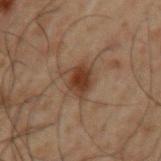Recorded during total-body skin imaging; not selected for excision or biopsy. This image is a 15 mm lesion crop taken from a total-body photograph. The lesion is on the left upper arm. The tile uses cross-polarized illumination. A male subject about 50 years old. An algorithmic analysis of the crop reported a nevus-likeness score of about 85/100 and a lesion-detection confidence of about 100/100.